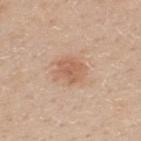Impression:
Captured during whole-body skin photography for melanoma surveillance; the lesion was not biopsied.
Background:
A male patient roughly 30 years of age. A 15 mm close-up extracted from a 3D total-body photography capture. Located on the back.